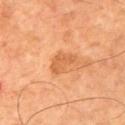Q: Was this lesion biopsied?
A: catalogued during a skin exam; not biopsied
Q: What kind of image is this?
A: 15 mm crop, total-body photography
Q: Lesion size?
A: ≈3.5 mm
Q: What is the anatomic site?
A: the left upper arm
Q: Who is the patient?
A: male, aged 43–47